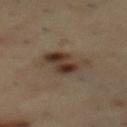Part of a total-body skin-imaging series; this lesion was reviewed on a skin check and was not flagged for biopsy.
The tile uses cross-polarized illumination.
A 15 mm close-up tile from a total-body photography series done for melanoma screening.
A male subject aged 53 to 57.
Located on the mid back.